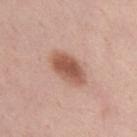Notes:
- workup: no biopsy performed (imaged during a skin exam)
- imaging modality: ~15 mm crop, total-body skin-cancer survey
- anatomic site: the chest
- patient: female, in their mid-30s
- lesion diameter: ≈5 mm
- tile lighting: white-light illumination